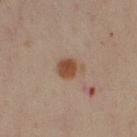This lesion was catalogued during total-body skin photography and was not selected for biopsy.
The recorded lesion diameter is about 3 mm.
Imaged with cross-polarized lighting.
The subject is a male aged approximately 50.
On the right upper arm.
A close-up tile cropped from a whole-body skin photograph, about 15 mm across.